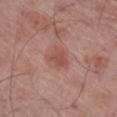| key | value |
|---|---|
| biopsy status | no biopsy performed (imaged during a skin exam) |
| lesion diameter | ~2.5 mm (longest diameter) |
| location | the right lower leg |
| image source | 15 mm crop, total-body photography |
| subject | male, roughly 70 years of age |
| automated lesion analysis | a lesion area of about 5 mm² and a symmetry-axis asymmetry near 0.25; roughly 8 lightness units darker than nearby skin and a normalized border contrast of about 6 |
| illumination | white-light illumination |